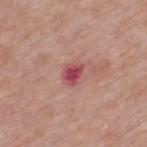A close-up tile cropped from a whole-body skin photograph, about 15 mm across. Captured under white-light illumination. The patient is a male aged around 55. Located on the upper back.